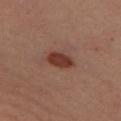This lesion was catalogued during total-body skin photography and was not selected for biopsy. The lesion is on the leg. The recorded lesion diameter is about 3.5 mm. A 15 mm crop from a total-body photograph taken for skin-cancer surveillance. The patient is a female in their 40s. Captured under cross-polarized illumination.